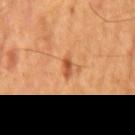Assessment: The lesion was photographed on a routine skin check and not biopsied; there is no pathology result. Background: Imaged with cross-polarized lighting. Located on the mid back. An algorithmic analysis of the crop reported a shape eccentricity near 0.9. The software also gave an automated nevus-likeness rating near 85 out of 100 and a lesion-detection confidence of about 100/100. A region of skin cropped from a whole-body photographic capture, roughly 15 mm wide. The recorded lesion diameter is about 2.5 mm. A male subject, aged 53–57.